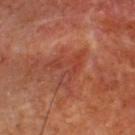Clinical impression:
Captured during whole-body skin photography for melanoma surveillance; the lesion was not biopsied.
Background:
A roughly 15 mm field-of-view crop from a total-body skin photograph. A male subject approximately 65 years of age. The lesion is located on the chest.